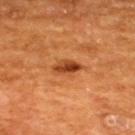The lesion was tiled from a total-body skin photograph and was not biopsied. Located on the upper back. A male subject in their mid-60s. The recorded lesion diameter is about 3.5 mm. The tile uses cross-polarized illumination. A close-up tile cropped from a whole-body skin photograph, about 15 mm across.